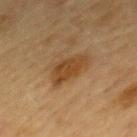Clinical impression:
No biopsy was performed on this lesion — it was imaged during a full skin examination and was not determined to be concerning.